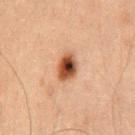The lesion was photographed on a routine skin check and not biopsied; there is no pathology result. This is a cross-polarized tile. A 15 mm close-up extracted from a 3D total-body photography capture. A male subject aged 48–52. Located on the abdomen. Longest diameter approximately 3.5 mm. Automated image analysis of the tile measured an area of roughly 7 mm², a shape eccentricity near 0.7, and a symmetry-axis asymmetry near 0.25. It also reported a mean CIELAB color near L≈38 a*≈19 b*≈28, about 15 CIELAB-L* units darker than the surrounding skin, and a normalized lesion–skin contrast near 12. The software also gave a color-variation rating of about 9.5/10 and peripheral color asymmetry of about 3. And it measured lesion-presence confidence of about 100/100.